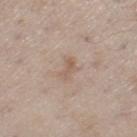The lesion was tiled from a total-body skin photograph and was not biopsied. This is a white-light tile. A male subject aged 58–62. Approximately 2.5 mm at its widest. The lesion-visualizer software estimated a lesion area of about 2.5 mm², an outline eccentricity of about 0.9 (0 = round, 1 = elongated), and two-axis asymmetry of about 0.5. From the left thigh. A 15 mm close-up tile from a total-body photography series done for melanoma screening.